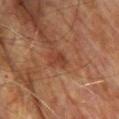follow-up: total-body-photography surveillance lesion; no biopsy.